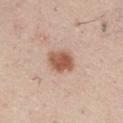biopsy status: total-body-photography surveillance lesion; no biopsy | anatomic site: the chest | image source: total-body-photography crop, ~15 mm field of view | image-analysis metrics: a border-irregularity rating of about 1.5/10, internal color variation of about 3.5 on a 0–10 scale, and a peripheral color-asymmetry measure near 1 | patient: male, aged around 60.